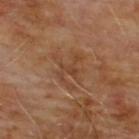Clinical impression:
Part of a total-body skin-imaging series; this lesion was reviewed on a skin check and was not flagged for biopsy.
Context:
A lesion tile, about 15 mm wide, cut from a 3D total-body photograph. On the chest. The patient is a male about 60 years old. The lesion's longest dimension is about 4.5 mm. This is a cross-polarized tile.